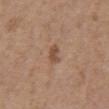The lesion was photographed on a routine skin check and not biopsied; there is no pathology result.
The lesion's longest dimension is about 2.5 mm.
The lesion is located on the abdomen.
The tile uses white-light illumination.
An algorithmic analysis of the crop reported a lesion area of about 3 mm² and two-axis asymmetry of about 0.3. The analysis additionally found an average lesion color of about L≈49 a*≈19 b*≈29 (CIELAB), roughly 10 lightness units darker than nearby skin, and a normalized border contrast of about 7.5. The analysis additionally found a nevus-likeness score of about 30/100 and a detector confidence of about 100 out of 100 that the crop contains a lesion.
A close-up tile cropped from a whole-body skin photograph, about 15 mm across.
A female subject, aged 63–67.